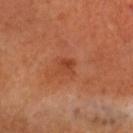The lesion was photographed on a routine skin check and not biopsied; there is no pathology result.